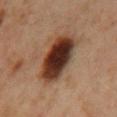The lesion was photographed on a routine skin check and not biopsied; there is no pathology result.
The lesion's longest dimension is about 6 mm.
A male subject, aged around 60.
A 15 mm crop from a total-body photograph taken for skin-cancer surveillance.
Captured under cross-polarized illumination.
On the mid back.
Automated tile analysis of the lesion measured two-axis asymmetry of about 0.1. And it measured a border-irregularity index near 2/10, a within-lesion color-variation index near 8.5/10, and peripheral color asymmetry of about 2.5.